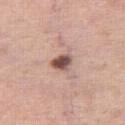biopsy status = total-body-photography surveillance lesion; no biopsy | patient = female, roughly 50 years of age | acquisition = ~15 mm crop, total-body skin-cancer survey | location = the right thigh | tile lighting = white-light.